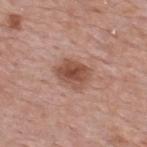Part of a total-body skin-imaging series; this lesion was reviewed on a skin check and was not flagged for biopsy. The tile uses white-light illumination. A lesion tile, about 15 mm wide, cut from a 3D total-body photograph. Located on the back. A male subject aged 53 to 57. The recorded lesion diameter is about 4 mm.